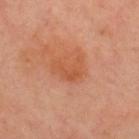Notes:
- follow-up — no biopsy performed (imaged during a skin exam)
- automated metrics — a lesion area of about 6.5 mm², a shape eccentricity near 0.75, and two-axis asymmetry of about 0.5; a nevus-likeness score of about 40/100 and a detector confidence of about 100 out of 100 that the crop contains a lesion
- illumination — cross-polarized illumination
- site — the upper back
- image source — ~15 mm crop, total-body skin-cancer survey
- patient — male, aged around 50